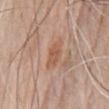Findings:
• biopsy status · catalogued during a skin exam; not biopsied
• subject · male, aged approximately 80
• tile lighting · white-light
• image-analysis metrics · a lesion area of about 6 mm², an eccentricity of roughly 0.85, and a symmetry-axis asymmetry near 0.2; a mean CIELAB color near L≈56 a*≈21 b*≈31, about 7 CIELAB-L* units darker than the surrounding skin, and a normalized lesion–skin contrast near 6.5; border irregularity of about 2.5 on a 0–10 scale, a color-variation rating of about 2/10, and radial color variation of about 0.5; an automated nevus-likeness rating near 0 out of 100 and a detector confidence of about 100 out of 100 that the crop contains a lesion
• size · ≈3.5 mm
• location · the chest
• image · total-body-photography crop, ~15 mm field of view